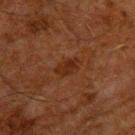Impression: The lesion was tiled from a total-body skin photograph and was not biopsied. Acquisition and patient details: A region of skin cropped from a whole-body photographic capture, roughly 15 mm wide. The lesion is located on the back. Approximately 3 mm at its widest. The tile uses cross-polarized illumination. A male subject, aged 58 to 62.